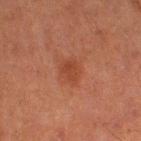  biopsy_status: not biopsied; imaged during a skin examination
  image:
    source: total-body photography crop
    field_of_view_mm: 15
  patient:
    sex: male
    age_approx: 65
  site: left thigh
  lighting: cross-polarized
  automated_metrics:
    border_irregularity_0_10: 2.5
    peripheral_color_asymmetry: 0.5
    nevus_likeness_0_100: 5
  lesion_size:
    long_diameter_mm_approx: 2.5